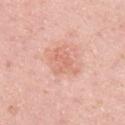{
  "biopsy_status": "not biopsied; imaged during a skin examination",
  "lesion_size": {
    "long_diameter_mm_approx": 4.0
  },
  "patient": {
    "sex": "female",
    "age_approx": 30
  },
  "image": {
    "source": "total-body photography crop",
    "field_of_view_mm": 15
  },
  "automated_metrics": {
    "cielab_L": 67,
    "cielab_a": 25,
    "cielab_b": 30,
    "vs_skin_darker_L": 8.0,
    "vs_skin_contrast_norm": 5.0,
    "color_variation_0_10": 2.5,
    "peripheral_color_asymmetry": 1.0,
    "nevus_likeness_0_100": 0
  },
  "lighting": "white-light",
  "site": "right upper arm"
}